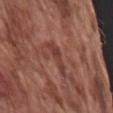Clinical impression:
No biopsy was performed on this lesion — it was imaged during a full skin examination and was not determined to be concerning.
Acquisition and patient details:
This is a white-light tile. The lesion is located on the arm. The lesion-visualizer software estimated an area of roughly 5.5 mm² and an eccentricity of roughly 0.9. It also reported a border-irregularity rating of about 6.5/10, a within-lesion color-variation index near 1.5/10, and radial color variation of about 0.5. A male subject aged around 75. The recorded lesion diameter is about 4.5 mm. A roughly 15 mm field-of-view crop from a total-body skin photograph.